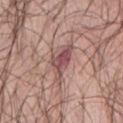Findings:
* workup: catalogued during a skin exam; not biopsied
* location: the abdomen
* lesion size: about 5 mm
* patient: female, in their mid-50s
* lighting: white-light illumination
* image: ~15 mm tile from a whole-body skin photo